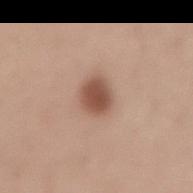| key | value |
|---|---|
| biopsy status | total-body-photography surveillance lesion; no biopsy |
| size | about 3 mm |
| location | the lower back |
| patient | male, in their mid- to late 50s |
| image source | ~15 mm tile from a whole-body skin photo |
| automated lesion analysis | a footprint of about 7 mm²; a mean CIELAB color near L≈52 a*≈20 b*≈28, roughly 14 lightness units darker than nearby skin, and a normalized lesion–skin contrast near 9.5; a border-irregularity index near 1.5/10 and internal color variation of about 2.5 on a 0–10 scale |
| tile lighting | white-light illumination |